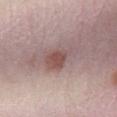Q: Is there a histopathology result?
A: total-body-photography surveillance lesion; no biopsy
Q: How was this image acquired?
A: ~15 mm tile from a whole-body skin photo
Q: What are the patient's age and sex?
A: male, about 50 years old
Q: Where on the body is the lesion?
A: the right lower leg